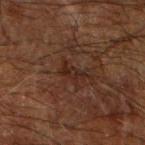| feature | finding |
|---|---|
| notes | total-body-photography surveillance lesion; no biopsy |
| image source | 15 mm crop, total-body photography |
| lighting | cross-polarized |
| anatomic site | the right forearm |
| lesion size | about 3 mm |
| patient | male, roughly 65 years of age |